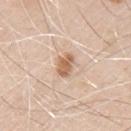The lesion was tiled from a total-body skin photograph and was not biopsied.
Automated tile analysis of the lesion measured an area of roughly 5 mm², an eccentricity of roughly 0.75, and two-axis asymmetry of about 0.25.
Longest diameter approximately 3 mm.
Captured under white-light illumination.
The subject is a male aged 68–72.
The lesion is on the arm.
A close-up tile cropped from a whole-body skin photograph, about 15 mm across.